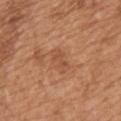The lesion was tiled from a total-body skin photograph and was not biopsied.
Located on the upper back.
The tile uses white-light illumination.
Automated tile analysis of the lesion measured an average lesion color of about L≈51 a*≈24 b*≈33 (CIELAB), a lesion–skin lightness drop of about 7, and a normalized lesion–skin contrast near 5. It also reported a border-irregularity rating of about 5/10, a color-variation rating of about 1.5/10, and peripheral color asymmetry of about 0.
A male patient, aged 63–67.
A roughly 15 mm field-of-view crop from a total-body skin photograph.